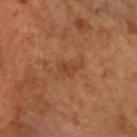Q: Was this lesion biopsied?
A: catalogued during a skin exam; not biopsied
Q: What did automated image analysis measure?
A: an area of roughly 3.5 mm² and a symmetry-axis asymmetry near 0.45; a border-irregularity index near 4.5/10, internal color variation of about 1.5 on a 0–10 scale, and a peripheral color-asymmetry measure near 0.5
Q: What is the anatomic site?
A: the right forearm
Q: What kind of image is this?
A: 15 mm crop, total-body photography
Q: Who is the patient?
A: female, in their 70s
Q: What is the lesion's diameter?
A: about 3 mm